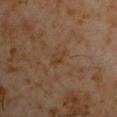<lesion>
  <biopsy_status>not biopsied; imaged during a skin examination</biopsy_status>
  <site>left upper arm</site>
  <image>
    <source>total-body photography crop</source>
    <field_of_view_mm>15</field_of_view_mm>
  </image>
  <patient>
    <sex>male</sex>
    <age_approx>60</age_approx>
  </patient>
  <automated_metrics>
    <area_mm2_approx>3.0</area_mm2_approx>
    <eccentricity>0.85</eccentricity>
    <shape_asymmetry>0.3</shape_asymmetry>
    <nevus_likeness_0_100>0</nevus_likeness_0_100>
    <lesion_detection_confidence_0_100>100</lesion_detection_confidence_0_100>
  </automated_metrics>
</lesion>